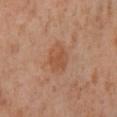{
  "biopsy_status": "not biopsied; imaged during a skin examination",
  "site": "right lower leg",
  "lesion_size": {
    "long_diameter_mm_approx": 4.0
  },
  "lighting": "cross-polarized",
  "automated_metrics": {
    "area_mm2_approx": 8.5,
    "eccentricity": 0.7,
    "shape_asymmetry": 0.15
  },
  "patient": {
    "sex": "female",
    "age_approx": 55
  },
  "image": {
    "source": "total-body photography crop",
    "field_of_view_mm": 15
  }
}